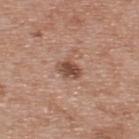Captured during whole-body skin photography for melanoma surveillance; the lesion was not biopsied. About 2.5 mm across. The subject is a male aged around 55. A 15 mm close-up extracted from a 3D total-body photography capture. Imaged with white-light lighting. The lesion is located on the upper back.